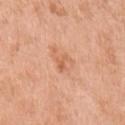The lesion was photographed on a routine skin check and not biopsied; there is no pathology result.
Captured under white-light illumination.
Cropped from a whole-body photographic skin survey; the tile spans about 15 mm.
On the left upper arm.
The lesion's longest dimension is about 2.5 mm.
Automated tile analysis of the lesion measured about 8 CIELAB-L* units darker than the surrounding skin and a normalized lesion–skin contrast near 5.5. The analysis additionally found a border-irregularity rating of about 4.5/10, internal color variation of about 1 on a 0–10 scale, and peripheral color asymmetry of about 0.5.
A female subject about 65 years old.